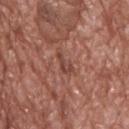biopsy_status: not biopsied; imaged during a skin examination
image:
  source: total-body photography crop
  field_of_view_mm: 15
patient:
  sex: male
  age_approx: 70
automated_metrics:
  area_mm2_approx: 3.0
  eccentricity: 0.95
  cielab_L: 44
  cielab_a: 24
  cielab_b: 26
  vs_skin_darker_L: 8.0
  vs_skin_contrast_norm: 6.5
  border_irregularity_0_10: 6.0
  lesion_detection_confidence_0_100: 75
site: upper back